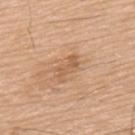Q: Is there a histopathology result?
A: catalogued during a skin exam; not biopsied
Q: Lesion location?
A: the upper back
Q: Lesion size?
A: ≈3.5 mm
Q: How was this image acquired?
A: total-body-photography crop, ~15 mm field of view
Q: Who is the patient?
A: male, about 80 years old